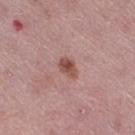Notes:
– biopsy status: imaged on a skin check; not biopsied
– patient: female, about 45 years old
– image source: 15 mm crop, total-body photography
– body site: the right thigh